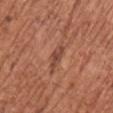Impression: Imaged during a routine full-body skin examination; the lesion was not biopsied and no histopathology is available. Context: Imaged with white-light lighting. On the left upper arm. About 3.5 mm across. A 15 mm close-up tile from a total-body photography series done for melanoma screening. A female subject, approximately 75 years of age.